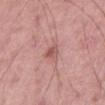biopsy status — total-body-photography surveillance lesion; no biopsy | lesion size — about 3 mm | image-analysis metrics — an outline eccentricity of about 0.8 (0 = round, 1 = elongated) and two-axis asymmetry of about 0.4; a mean CIELAB color near L≈55 a*≈24 b*≈24 and a lesion–skin lightness drop of about 9; a border-irregularity index near 3.5/10 and internal color variation of about 3.5 on a 0–10 scale; a classifier nevus-likeness of about 0/100 and a lesion-detection confidence of about 100/100 | lighting — white-light illumination | site — the leg | subject — male, aged 53 to 57 | imaging modality — ~15 mm tile from a whole-body skin photo.